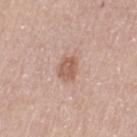Case summary:
• workup — imaged on a skin check; not biopsied
• subject — female, aged around 70
• lighting — white-light illumination
• automated metrics — two-axis asymmetry of about 0.25; a border-irregularity index near 2.5/10, a within-lesion color-variation index near 2/10, and radial color variation of about 1; a classifier nevus-likeness of about 35/100 and a detector confidence of about 100 out of 100 that the crop contains a lesion
• site — the left thigh
• image — 15 mm crop, total-body photography